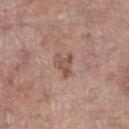Q: Was a biopsy performed?
A: no biopsy performed (imaged during a skin exam)
Q: What lighting was used for the tile?
A: white-light illumination
Q: What is the lesion's diameter?
A: ≈3 mm
Q: Automated lesion metrics?
A: an area of roughly 4.5 mm², an outline eccentricity of about 0.7 (0 = round, 1 = elongated), and a shape-asymmetry score of about 0.45 (0 = symmetric); about 9 CIELAB-L* units darker than the surrounding skin and a normalized lesion–skin contrast near 6.5; a peripheral color-asymmetry measure near 0.5
Q: What kind of image is this?
A: 15 mm crop, total-body photography
Q: Lesion location?
A: the left lower leg
Q: Who is the patient?
A: female, in their mid- to late 80s or older